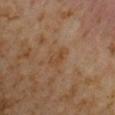notes — imaged on a skin check; not biopsied
acquisition — 15 mm crop, total-body photography
lesion diameter — about 3 mm
subject — male, aged 58–62
lighting — cross-polarized illumination
body site — the arm